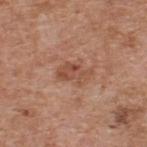follow-up: imaged on a skin check; not biopsied
tile lighting: white-light
lesion size: ~4 mm (longest diameter)
image: 15 mm crop, total-body photography
site: the back
subject: male, approximately 60 years of age